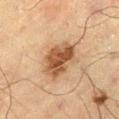Q: How large is the lesion?
A: ~4.5 mm (longest diameter)
Q: What did automated image analysis measure?
A: internal color variation of about 4 on a 0–10 scale and peripheral color asymmetry of about 1.5
Q: What kind of image is this?
A: 15 mm crop, total-body photography
Q: Who is the patient?
A: male, about 70 years old
Q: Lesion location?
A: the left thigh